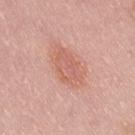Clinical impression: Captured during whole-body skin photography for melanoma surveillance; the lesion was not biopsied. Background: A roughly 15 mm field-of-view crop from a total-body skin photograph. The patient is a male in their 40s. Automated tile analysis of the lesion measured a lesion color around L≈62 a*≈26 b*≈29 in CIELAB, roughly 8 lightness units darker than nearby skin, and a normalized lesion–skin contrast near 5.5. It also reported a border-irregularity index near 2.5/10, a within-lesion color-variation index near 3.5/10, and radial color variation of about 1.5. And it measured a nevus-likeness score of about 85/100 and a lesion-detection confidence of about 100/100. The recorded lesion diameter is about 5.5 mm. Captured under white-light illumination. From the lower back.